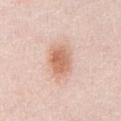Assessment: Imaged during a routine full-body skin examination; the lesion was not biopsied and no histopathology is available. Background: The total-body-photography lesion software estimated about 12 CIELAB-L* units darker than the surrounding skin and a lesion-to-skin contrast of about 8 (normalized; higher = more distinct). It also reported a nevus-likeness score of about 100/100. Approximately 4 mm at its widest. A region of skin cropped from a whole-body photographic capture, roughly 15 mm wide. Imaged with white-light lighting. On the chest. A male subject aged 23 to 27.